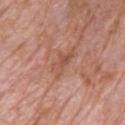Impression: No biopsy was performed on this lesion — it was imaged during a full skin examination and was not determined to be concerning. Clinical summary: The tile uses white-light illumination. Automated tile analysis of the lesion measured a lesion color around L≈53 a*≈24 b*≈30 in CIELAB, about 7 CIELAB-L* units darker than the surrounding skin, and a normalized border contrast of about 5.5. The analysis additionally found a color-variation rating of about 3.5/10 and radial color variation of about 1. A 15 mm close-up tile from a total-body photography series done for melanoma screening. Located on the chest. The recorded lesion diameter is about 4.5 mm. A male subject, aged approximately 80.